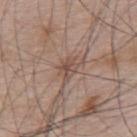| key | value |
|---|---|
| follow-up | catalogued during a skin exam; not biopsied |
| subject | male, roughly 65 years of age |
| lesion size | ~2.5 mm (longest diameter) |
| TBP lesion metrics | a footprint of about 3.5 mm² and a shape-asymmetry score of about 0.35 (0 = symmetric) |
| acquisition | total-body-photography crop, ~15 mm field of view |
| lighting | white-light illumination |
| site | the upper back |